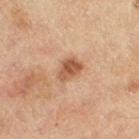Captured during whole-body skin photography for melanoma surveillance; the lesion was not biopsied. The lesion is located on the right thigh. The lesion-visualizer software estimated internal color variation of about 3.5 on a 0–10 scale and a peripheral color-asymmetry measure near 1.5. And it measured a nevus-likeness score of about 80/100 and a detector confidence of about 100 out of 100 that the crop contains a lesion. A roughly 15 mm field-of-view crop from a total-body skin photograph. The lesion's longest dimension is about 3 mm. A female subject, aged 53–57.